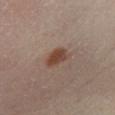workup: imaged on a skin check; not biopsied
subject: female, aged 38 to 42
imaging modality: 15 mm crop, total-body photography
illumination: cross-polarized illumination
location: the right leg